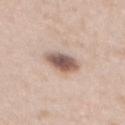On the back. A 15 mm close-up extracted from a 3D total-body photography capture. Automated tile analysis of the lesion measured a lesion color around L≈56 a*≈17 b*≈23 in CIELAB and roughly 17 lightness units darker than nearby skin. A female patient roughly 30 years of age.